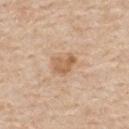Part of a total-body skin-imaging series; this lesion was reviewed on a skin check and was not flagged for biopsy. The total-body-photography lesion software estimated a mean CIELAB color near L≈60 a*≈19 b*≈35. It also reported a border-irregularity index near 3/10, internal color variation of about 2 on a 0–10 scale, and a peripheral color-asymmetry measure near 0.5. The software also gave a classifier nevus-likeness of about 15/100 and a lesion-detection confidence of about 100/100. A 15 mm crop from a total-body photograph taken for skin-cancer surveillance. This is a white-light tile. From the mid back. Longest diameter approximately 3 mm. A male patient aged 53 to 57.